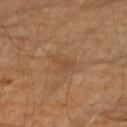The lesion was tiled from a total-body skin photograph and was not biopsied. This image is a 15 mm lesion crop taken from a total-body photograph. Located on the right upper arm. A male patient about 60 years old. Captured under cross-polarized illumination.